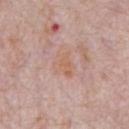biopsy_status: not biopsied; imaged during a skin examination
image:
  source: total-body photography crop
  field_of_view_mm: 15
lighting: white-light
site: chest
patient:
  sex: male
  age_approx: 70
automated_metrics:
  shape_asymmetry: 0.4
  vs_skin_contrast_norm: 5.5
  border_irregularity_0_10: 4.0
  color_variation_0_10: 2.0
  nevus_likeness_0_100: 0
lesion_size:
  long_diameter_mm_approx: 3.0